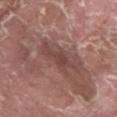Imaged during a routine full-body skin examination; the lesion was not biopsied and no histopathology is available.
From the left thigh.
Automated tile analysis of the lesion measured a lesion area of about 8 mm², a shape eccentricity near 0.85, and a shape-asymmetry score of about 0.4 (0 = symmetric). And it measured border irregularity of about 5.5 on a 0–10 scale, a within-lesion color-variation index near 2/10, and peripheral color asymmetry of about 0.5. It also reported a classifier nevus-likeness of about 0/100.
Longest diameter approximately 4.5 mm.
A male patient in their 40s.
A roughly 15 mm field-of-view crop from a total-body skin photograph.